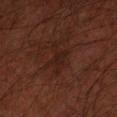Case summary:
• notes · total-body-photography surveillance lesion; no biopsy
• acquisition · ~15 mm tile from a whole-body skin photo
• TBP lesion metrics · a border-irregularity index near 6.5/10, internal color variation of about 1 on a 0–10 scale, and a peripheral color-asymmetry measure near 0.5; a classifier nevus-likeness of about 0/100 and a lesion-detection confidence of about 95/100
• site · the left forearm
• patient · male, in their 60s